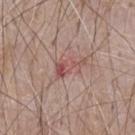* workup: imaged on a skin check; not biopsied
* imaging modality: ~15 mm tile from a whole-body skin photo
* automated metrics: a lesion–skin lightness drop of about 9 and a normalized lesion–skin contrast near 6; a border-irregularity index near 6.5/10 and a within-lesion color-variation index near 5/10; a classifier nevus-likeness of about 0/100 and a detector confidence of about 100 out of 100 that the crop contains a lesion
* subject: male, about 65 years old
* anatomic site: the chest
* diameter: ~4.5 mm (longest diameter)
* lighting: white-light